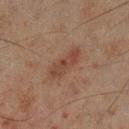biopsy_status: not biopsied; imaged during a skin examination
image:
  source: total-body photography crop
  field_of_view_mm: 15
patient:
  sex: male
  age_approx: 45
site: left lower leg